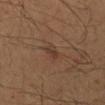The lesion was tiled from a total-body skin photograph and was not biopsied. Approximately 3 mm at its widest. Cropped from a total-body skin-imaging series; the visible field is about 15 mm. On the mid back. The tile uses cross-polarized illumination. An algorithmic analysis of the crop reported an area of roughly 3 mm² and a shape eccentricity near 0.9. The software also gave a lesion color around L≈35 a*≈17 b*≈25 in CIELAB and a lesion–skin lightness drop of about 6. The software also gave border irregularity of about 3 on a 0–10 scale, a within-lesion color-variation index near 0/10, and a peripheral color-asymmetry measure near 0. The subject is a male about 50 years old.